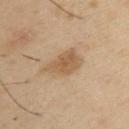Impression:
Captured during whole-body skin photography for melanoma surveillance; the lesion was not biopsied.
Background:
This image is a 15 mm lesion crop taken from a total-body photograph. The lesion is on the upper back. The subject is a male about 40 years old.